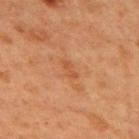<lesion>
  <biopsy_status>not biopsied; imaged during a skin examination</biopsy_status>
  <image>
    <source>total-body photography crop</source>
    <field_of_view_mm>15</field_of_view_mm>
  </image>
  <site>mid back</site>
  <patient>
    <sex>female</sex>
    <age_approx>40</age_approx>
  </patient>
</lesion>